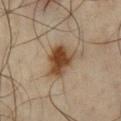TBP lesion metrics: a lesion area of about 10 mm², a shape eccentricity near 0.7, and a shape-asymmetry score of about 0.25 (0 = symmetric); an automated nevus-likeness rating near 100 out of 100 and a lesion-detection confidence of about 100/100
lighting: cross-polarized
patient: male, about 65 years old
anatomic site: the chest
imaging modality: ~15 mm tile from a whole-body skin photo